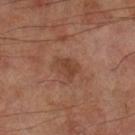The lesion was tiled from a total-body skin photograph and was not biopsied.
Longest diameter approximately 3 mm.
Captured under cross-polarized illumination.
The lesion is located on the left lower leg.
A male patient aged 68 to 72.
A close-up tile cropped from a whole-body skin photograph, about 15 mm across.